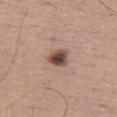Assessment: No biopsy was performed on this lesion — it was imaged during a full skin examination and was not determined to be concerning. Image and clinical context: Located on the left thigh. The patient is a male aged 58–62. This image is a 15 mm lesion crop taken from a total-body photograph. Imaged with white-light lighting. The recorded lesion diameter is about 2.5 mm.